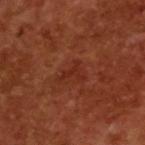No biopsy was performed on this lesion — it was imaged during a full skin examination and was not determined to be concerning. The tile uses cross-polarized illumination. The recorded lesion diameter is about 3 mm. A 15 mm close-up extracted from a 3D total-body photography capture. A male subject aged 63–67.